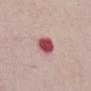The lesion was photographed on a routine skin check and not biopsied; there is no pathology result. Imaged with white-light lighting. Automated image analysis of the tile measured an eccentricity of roughly 0.65. And it measured a peripheral color-asymmetry measure near 1. It also reported a nevus-likeness score of about 0/100 and a detector confidence of about 100 out of 100 that the crop contains a lesion. A male patient in their 50s. The lesion is on the abdomen. The recorded lesion diameter is about 3 mm. Cropped from a total-body skin-imaging series; the visible field is about 15 mm.